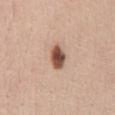On the abdomen. A roughly 15 mm field-of-view crop from a total-body skin photograph. The subject is a female aged 38–42. This is a white-light tile. An algorithmic analysis of the crop reported an area of roughly 5.5 mm², an outline eccentricity of about 0.8 (0 = round, 1 = elongated), and two-axis asymmetry of about 0.15. It also reported a within-lesion color-variation index near 5/10 and radial color variation of about 1.5. Approximately 3.5 mm at its widest.